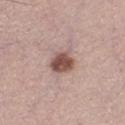notes: imaged on a skin check; not biopsied
lesion diameter: about 3 mm
automated lesion analysis: an area of roughly 6.5 mm² and an eccentricity of roughly 0.4; a lesion color around L≈50 a*≈20 b*≈23 in CIELAB, a lesion–skin lightness drop of about 16, and a lesion-to-skin contrast of about 10.5 (normalized; higher = more distinct); a border-irregularity index near 2/10, internal color variation of about 4 on a 0–10 scale, and a peripheral color-asymmetry measure near 1.5
subject: male, aged approximately 30
acquisition: ~15 mm crop, total-body skin-cancer survey